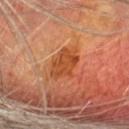Captured during whole-body skin photography for melanoma surveillance; the lesion was not biopsied. A roughly 15 mm field-of-view crop from a total-body skin photograph. A male subject, aged around 65. The recorded lesion diameter is about 5 mm. On the head or neck. Captured under cross-polarized illumination.